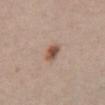biopsy_status: not biopsied; imaged during a skin examination
patient:
  sex: male
  age_approx: 40
image:
  source: total-body photography crop
  field_of_view_mm: 15
lighting: white-light
lesion_size:
  long_diameter_mm_approx: 2.5
site: abdomen